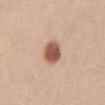Captured during whole-body skin photography for melanoma surveillance; the lesion was not biopsied.
About 3 mm across.
This is a white-light tile.
A female patient, about 25 years old.
A 15 mm close-up tile from a total-body photography series done for melanoma screening.
The lesion is on the abdomen.